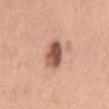biopsy status: catalogued during a skin exam; not biopsied | location: the mid back | subject: female, in their 40s | image source: ~15 mm crop, total-body skin-cancer survey | image-analysis metrics: a lesion area of about 7.5 mm² and an eccentricity of roughly 0.75; a color-variation rating of about 6.5/10 and peripheral color asymmetry of about 2.